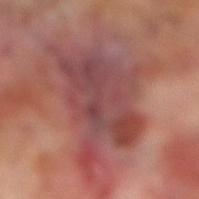Assessment:
The lesion was photographed on a routine skin check and not biopsied; there is no pathology result.
Acquisition and patient details:
Measured at roughly 10.5 mm in maximum diameter. A 15 mm crop from a total-body photograph taken for skin-cancer surveillance. A male patient aged approximately 70. Captured under cross-polarized illumination. Located on the leg.